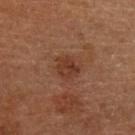site = the left arm
subject = female, approximately 65 years of age
lesion size = about 3 mm
acquisition = ~15 mm crop, total-body skin-cancer survey
illumination = cross-polarized illumination